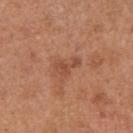| key | value |
|---|---|
| follow-up | total-body-photography surveillance lesion; no biopsy |
| image | ~15 mm crop, total-body skin-cancer survey |
| TBP lesion metrics | a lesion color around L≈49 a*≈24 b*≈32 in CIELAB and a normalized lesion–skin contrast near 6; a border-irregularity rating of about 6.5/10, a within-lesion color-variation index near 1/10, and radial color variation of about 0.5; a classifier nevus-likeness of about 0/100 and a detector confidence of about 100 out of 100 that the crop contains a lesion |
| tile lighting | white-light illumination |
| body site | the left upper arm |
| subject | female, aged approximately 30 |
| size | ~3 mm (longest diameter) |